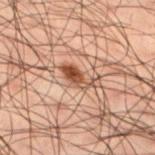Assessment:
The lesion was tiled from a total-body skin photograph and was not biopsied.
Background:
The lesion is on the left lower leg. A region of skin cropped from a whole-body photographic capture, roughly 15 mm wide. Longest diameter approximately 4 mm. A male patient, about 50 years old. The tile uses cross-polarized illumination.